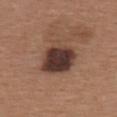| feature | finding |
|---|---|
| follow-up | catalogued during a skin exam; not biopsied |
| imaging modality | ~15 mm tile from a whole-body skin photo |
| patient | female, aged around 40 |
| location | the back |
| lighting | white-light illumination |